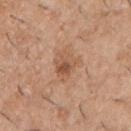notes — no biopsy performed (imaged during a skin exam) | body site — the chest | tile lighting — white-light illumination | patient — male, aged 38–42 | size — about 3.5 mm | automated metrics — a lesion area of about 7.5 mm², an eccentricity of roughly 0.65, and a shape-asymmetry score of about 0.25 (0 = symmetric); border irregularity of about 3 on a 0–10 scale, internal color variation of about 6 on a 0–10 scale, and a peripheral color-asymmetry measure near 2 | imaging modality — ~15 mm tile from a whole-body skin photo.